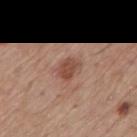Clinical impression: No biopsy was performed on this lesion — it was imaged during a full skin examination and was not determined to be concerning. Background: A male subject aged around 75. Located on the back. This is a white-light tile. A lesion tile, about 15 mm wide, cut from a 3D total-body photograph.